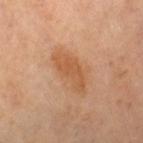The lesion was photographed on a routine skin check and not biopsied; there is no pathology result. Automated tile analysis of the lesion measured a footprint of about 8.5 mm², an outline eccentricity of about 0.9 (0 = round, 1 = elongated), and a symmetry-axis asymmetry near 0.4. It also reported roughly 8 lightness units darker than nearby skin and a normalized lesion–skin contrast near 6.5. A region of skin cropped from a whole-body photographic capture, roughly 15 mm wide. The tile uses cross-polarized illumination. From the right thigh. Approximately 5 mm at its widest. A female patient approximately 50 years of age.